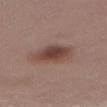workup: catalogued during a skin exam; not biopsied
imaging modality: 15 mm crop, total-body photography
tile lighting: white-light
lesion diameter: ~4 mm (longest diameter)
automated lesion analysis: an automated nevus-likeness rating near 95 out of 100 and lesion-presence confidence of about 100/100
anatomic site: the abdomen
subject: female, approximately 55 years of age